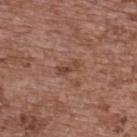Imaged during a routine full-body skin examination; the lesion was not biopsied and no histopathology is available. From the upper back. Measured at roughly 3 mm in maximum diameter. A 15 mm close-up tile from a total-body photography series done for melanoma screening. A female subject in their mid-60s.